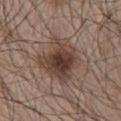notes: imaged on a skin check; not biopsied | tile lighting: white-light illumination | lesion size: ~5.5 mm (longest diameter) | acquisition: ~15 mm tile from a whole-body skin photo | automated lesion analysis: an outline eccentricity of about 0.45 (0 = round, 1 = elongated) and a shape-asymmetry score of about 0.25 (0 = symmetric); a within-lesion color-variation index near 6.5/10 and radial color variation of about 2; a classifier nevus-likeness of about 95/100 and a lesion-detection confidence of about 100/100 | subject: male, approximately 65 years of age | location: the chest.